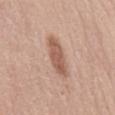The lesion was photographed on a routine skin check and not biopsied; there is no pathology result.
A 15 mm crop from a total-body photograph taken for skin-cancer surveillance.
Located on the mid back.
The recorded lesion diameter is about 5 mm.
Imaged with white-light lighting.
The lesion-visualizer software estimated an average lesion color of about L≈57 a*≈20 b*≈28 (CIELAB), about 12 CIELAB-L* units darker than the surrounding skin, and a lesion-to-skin contrast of about 8 (normalized; higher = more distinct). And it measured a nevus-likeness score of about 80/100 and a detector confidence of about 100 out of 100 that the crop contains a lesion.
A female patient, about 50 years old.